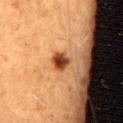notes: total-body-photography surveillance lesion; no biopsy | patient: male, roughly 65 years of age | tile lighting: cross-polarized illumination | TBP lesion metrics: an average lesion color of about L≈41 a*≈26 b*≈36 (CIELAB) and roughly 17 lightness units darker than nearby skin; lesion-presence confidence of about 100/100 | site: the lower back | lesion size: ≈2.5 mm | image: total-body-photography crop, ~15 mm field of view.